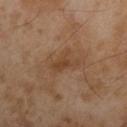Captured during whole-body skin photography for melanoma surveillance; the lesion was not biopsied.
On the left upper arm.
Cropped from a total-body skin-imaging series; the visible field is about 15 mm.
A male subject, approximately 55 years of age.